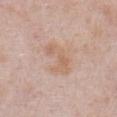Impression: This lesion was catalogued during total-body skin photography and was not selected for biopsy. Acquisition and patient details: The subject is a male aged approximately 55. Longest diameter approximately 5 mm. This is a white-light tile. On the front of the torso. This image is a 15 mm lesion crop taken from a total-body photograph.